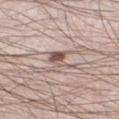Notes:
* follow-up — imaged on a skin check; not biopsied
* site — the left thigh
* patient — male, aged 33–37
* image — total-body-photography crop, ~15 mm field of view
* lesion size — about 4 mm